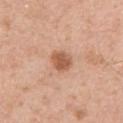The lesion was photographed on a routine skin check and not biopsied; there is no pathology result. A roughly 15 mm field-of-view crop from a total-body skin photograph. From the right upper arm. An algorithmic analysis of the crop reported a lesion–skin lightness drop of about 12 and a normalized lesion–skin contrast near 8. It also reported a classifier nevus-likeness of about 90/100. A male subject in their mid-50s. About 3 mm across. The tile uses white-light illumination.